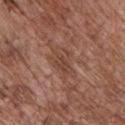* tile lighting · white-light
* diameter · about 4 mm
* patient · male, aged 68–72
* image source · total-body-photography crop, ~15 mm field of view
* site · the chest